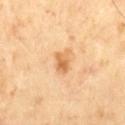Notes:
* notes — no biopsy performed (imaged during a skin exam)
* imaging modality — ~15 mm tile from a whole-body skin photo
* diameter — ≈3 mm
* image-analysis metrics — a lesion area of about 4.5 mm², an eccentricity of roughly 0.7, and two-axis asymmetry of about 0.25; a mean CIELAB color near L≈65 a*≈22 b*≈43, about 11 CIELAB-L* units darker than the surrounding skin, and a normalized lesion–skin contrast near 7.5; a border-irregularity index near 2.5/10 and a within-lesion color-variation index near 4/10; a classifier nevus-likeness of about 20/100
* patient — male, aged approximately 65